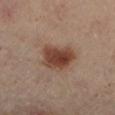Recorded during total-body skin imaging; not selected for excision or biopsy.
The lesion is located on the left lower leg.
An algorithmic analysis of the crop reported a lesion–skin lightness drop of about 12 and a normalized border contrast of about 10. The analysis additionally found a border-irregularity index near 2.5/10, internal color variation of about 5 on a 0–10 scale, and a peripheral color-asymmetry measure near 1.5. The software also gave a nevus-likeness score of about 100/100 and a lesion-detection confidence of about 100/100.
A female patient aged around 60.
Cropped from a whole-body photographic skin survey; the tile spans about 15 mm.
The recorded lesion diameter is about 4.5 mm.
The tile uses cross-polarized illumination.